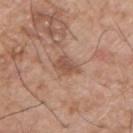Assessment:
The lesion was photographed on a routine skin check and not biopsied; there is no pathology result.
Acquisition and patient details:
A lesion tile, about 15 mm wide, cut from a 3D total-body photograph. A male patient, approximately 75 years of age. From the chest.